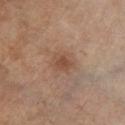notes: catalogued during a skin exam; not biopsied
patient: male, in their mid-60s
size: about 3 mm
acquisition: total-body-photography crop, ~15 mm field of view
tile lighting: cross-polarized illumination
body site: the left lower leg